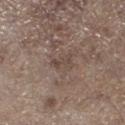Q: Was a biopsy performed?
A: no biopsy performed (imaged during a skin exam)
Q: Where on the body is the lesion?
A: the leg
Q: What is the imaging modality?
A: ~15 mm tile from a whole-body skin photo
Q: What lighting was used for the tile?
A: white-light illumination
Q: Automated lesion metrics?
A: a shape-asymmetry score of about 0.4 (0 = symmetric); a lesion color around L≈44 a*≈14 b*≈22 in CIELAB and a lesion-to-skin contrast of about 5.5 (normalized; higher = more distinct); a within-lesion color-variation index near 0/10; a nevus-likeness score of about 0/100
Q: What are the patient's age and sex?
A: male, aged 68–72